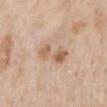– acquisition · ~15 mm tile from a whole-body skin photo
– site · the mid back
– subject · male, roughly 60 years of age